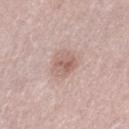Background:
The total-body-photography lesion software estimated a footprint of about 6 mm², an outline eccentricity of about 0.55 (0 = round, 1 = elongated), and two-axis asymmetry of about 0.2. A 15 mm close-up tile from a total-body photography series done for melanoma screening. Captured under white-light illumination. The recorded lesion diameter is about 3 mm. A female patient, aged 53 to 57. The lesion is on the leg.